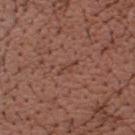Imaged during a routine full-body skin examination; the lesion was not biopsied and no histopathology is available.
A male subject, approximately 65 years of age.
A region of skin cropped from a whole-body photographic capture, roughly 15 mm wide.
From the head or neck.